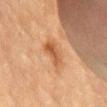Q: Automated lesion metrics?
A: an area of roughly 5.5 mm², an eccentricity of roughly 0.85, and a shape-asymmetry score of about 0.25 (0 = symmetric); radial color variation of about 1.5; an automated nevus-likeness rating near 0 out of 100
Q: Who is the patient?
A: male, approximately 85 years of age or older
Q: Where on the body is the lesion?
A: the mid back
Q: What kind of image is this?
A: 15 mm crop, total-body photography
Q: What lighting was used for the tile?
A: cross-polarized